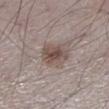Clinical impression: Part of a total-body skin-imaging series; this lesion was reviewed on a skin check and was not flagged for biopsy. Context: The lesion-visualizer software estimated an area of roughly 8 mm², an outline eccentricity of about 0.55 (0 = round, 1 = elongated), and two-axis asymmetry of about 0.15. From the left lower leg. The patient is a male aged 68 to 72. About 3.5 mm across. Imaged with white-light lighting. Cropped from a whole-body photographic skin survey; the tile spans about 15 mm.